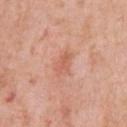Recorded during total-body skin imaging; not selected for excision or biopsy.
The lesion is on the chest.
The subject is a male roughly 60 years of age.
The tile uses white-light illumination.
A roughly 15 mm field-of-view crop from a total-body skin photograph.